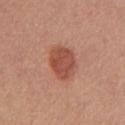No biopsy was performed on this lesion — it was imaged during a full skin examination and was not determined to be concerning. The lesion's longest dimension is about 4 mm. A 15 mm crop from a total-body photograph taken for skin-cancer surveillance. On the chest. The patient is a female aged approximately 55. This is a white-light tile.